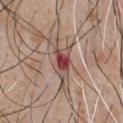follow-up = no biopsy performed (imaged during a skin exam) | illumination = white-light illumination | site = the chest | image = 15 mm crop, total-body photography | lesion diameter = ≈6.5 mm | TBP lesion metrics = an average lesion color of about L≈50 a*≈20 b*≈23 (CIELAB); a border-irregularity rating of about 7/10, a color-variation rating of about 7.5/10, and a peripheral color-asymmetry measure near 1.5; a classifier nevus-likeness of about 0/100 | subject = male, aged approximately 50.